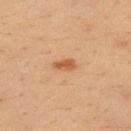Impression: Captured during whole-body skin photography for melanoma surveillance; the lesion was not biopsied. Clinical summary: Captured under cross-polarized illumination. The lesion is located on the upper back. The recorded lesion diameter is about 2.5 mm. A roughly 15 mm field-of-view crop from a total-body skin photograph. A male subject aged approximately 35.